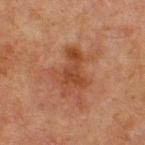<record>
<biopsy_status>not biopsied; imaged during a skin examination</biopsy_status>
<lesion_size>
  <long_diameter_mm_approx>5.0</long_diameter_mm_approx>
</lesion_size>
<image>
  <source>total-body photography crop</source>
  <field_of_view_mm>15</field_of_view_mm>
</image>
<patient>
  <sex>male</sex>
  <age_approx>65</age_approx>
</patient>
<lighting>cross-polarized</lighting>
<site>chest</site>
</record>